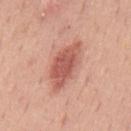Assessment:
Imaged during a routine full-body skin examination; the lesion was not biopsied and no histopathology is available.